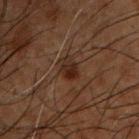Image and clinical context:
A male patient roughly 50 years of age. About 3 mm across. A lesion tile, about 15 mm wide, cut from a 3D total-body photograph. The lesion is on the upper back. The total-body-photography lesion software estimated an area of roughly 4.5 mm² and a shape eccentricity near 0.75. And it measured a lesion color around L≈19 a*≈14 b*≈20 in CIELAB, a lesion–skin lightness drop of about 7, and a normalized border contrast of about 9. Imaged with cross-polarized lighting.